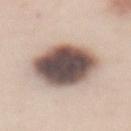Part of a total-body skin-imaging series; this lesion was reviewed on a skin check and was not flagged for biopsy. A lesion tile, about 15 mm wide, cut from a 3D total-body photograph. Located on the mid back. A female patient about 50 years old.